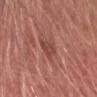Captured during whole-body skin photography for melanoma surveillance; the lesion was not biopsied. Captured under white-light illumination. About 3 mm across. A 15 mm crop from a total-body photograph taken for skin-cancer surveillance. The patient is a female aged approximately 40. On the head or neck.